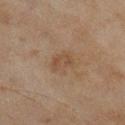Impression:
No biopsy was performed on this lesion — it was imaged during a full skin examination and was not determined to be concerning.
Background:
Located on the right lower leg. A female subject roughly 60 years of age. An algorithmic analysis of the crop reported a lesion area of about 5 mm² and an outline eccentricity of about 0.75 (0 = round, 1 = elongated). The analysis additionally found a mean CIELAB color near L≈44 a*≈15 b*≈28 and a lesion–skin lightness drop of about 6. The software also gave a classifier nevus-likeness of about 0/100. Measured at roughly 3 mm in maximum diameter. Cropped from a total-body skin-imaging series; the visible field is about 15 mm.